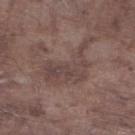follow-up=catalogued during a skin exam; not biopsied
subject=male, in their mid-70s
lesion diameter=about 7.5 mm
image source=~15 mm tile from a whole-body skin photo
site=the right lower leg